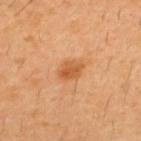The lesion is on the upper back.
Cropped from a whole-body photographic skin survey; the tile spans about 15 mm.
About 3 mm across.
A male subject, about 30 years old.
Automated tile analysis of the lesion measured an eccentricity of roughly 0.7. The analysis additionally found a classifier nevus-likeness of about 80/100 and a detector confidence of about 100 out of 100 that the crop contains a lesion.
The tile uses cross-polarized illumination.